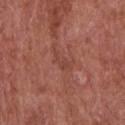Captured during whole-body skin photography for melanoma surveillance; the lesion was not biopsied.
A lesion tile, about 15 mm wide, cut from a 3D total-body photograph.
The patient is a male aged around 65.
The lesion's longest dimension is about 3 mm.
The lesion-visualizer software estimated a lesion area of about 3.5 mm², an outline eccentricity of about 0.9 (0 = round, 1 = elongated), and two-axis asymmetry of about 0.55. The analysis additionally found a lesion–skin lightness drop of about 6 and a normalized border contrast of about 4.5.
The lesion is on the chest.